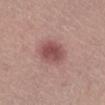Case summary:
* notes — imaged on a skin check; not biopsied
* site — the right lower leg
* imaging modality — total-body-photography crop, ~15 mm field of view
* patient — female, about 20 years old
* lighting — white-light